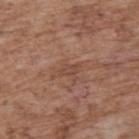No biopsy was performed on this lesion — it was imaged during a full skin examination and was not determined to be concerning.
The lesion is located on the upper back.
A region of skin cropped from a whole-body photographic capture, roughly 15 mm wide.
An algorithmic analysis of the crop reported border irregularity of about 4 on a 0–10 scale and internal color variation of about 0.5 on a 0–10 scale.
A male subject aged approximately 70.
About 2.5 mm across.
The tile uses white-light illumination.